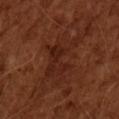<tbp_lesion>
  <biopsy_status>not biopsied; imaged during a skin examination</biopsy_status>
  <automated_metrics>
    <area_mm2_approx>17.0</area_mm2_approx>
    <eccentricity>0.85</eccentricity>
    <shape_asymmetry>0.35</shape_asymmetry>
    <vs_skin_darker_L>5.0</vs_skin_darker_L>
    <vs_skin_contrast_norm>6.0</vs_skin_contrast_norm>
    <nevus_likeness_0_100>10</nevus_likeness_0_100>
  </automated_metrics>
  <lesion_size>
    <long_diameter_mm_approx>6.5</long_diameter_mm_approx>
  </lesion_size>
  <patient>
    <sex>male</sex>
    <age_approx>65</age_approx>
  </patient>
  <lighting>cross-polarized</lighting>
  <image>
    <source>total-body photography crop</source>
    <field_of_view_mm>15</field_of_view_mm>
  </image>
</tbp_lesion>